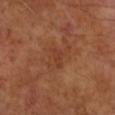Assessment:
Imaged during a routine full-body skin examination; the lesion was not biopsied and no histopathology is available.
Background:
The tile uses cross-polarized illumination. Automated image analysis of the tile measured a footprint of about 3 mm², an eccentricity of roughly 0.85, and a shape-asymmetry score of about 0.35 (0 = symmetric). It also reported roughly 5 lightness units darker than nearby skin and a normalized lesion–skin contrast near 4.5. The analysis additionally found a lesion-detection confidence of about 100/100. The recorded lesion diameter is about 3 mm. A male patient about 55 years old. From the right forearm. A 15 mm crop from a total-body photograph taken for skin-cancer surveillance.